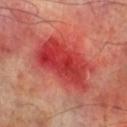Clinical impression:
The lesion was photographed on a routine skin check and not biopsied; there is no pathology result.
Clinical summary:
The total-body-photography lesion software estimated an area of roughly 28 mm² and a shape eccentricity near 0.85. The software also gave an average lesion color of about L≈44 a*≈44 b*≈32 (CIELAB), about 12 CIELAB-L* units darker than the surrounding skin, and a normalized lesion–skin contrast near 8.5. And it measured border irregularity of about 3 on a 0–10 scale and a color-variation rating of about 6.5/10. The tile uses cross-polarized illumination. Measured at roughly 8 mm in maximum diameter. The lesion is located on the right lower leg. A male patient roughly 70 years of age. A roughly 15 mm field-of-view crop from a total-body skin photograph.